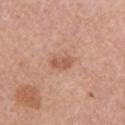{"biopsy_status": "not biopsied; imaged during a skin examination", "patient": {"sex": "female", "age_approx": 60}, "lighting": "white-light", "image": {"source": "total-body photography crop", "field_of_view_mm": 15}, "site": "right upper arm"}